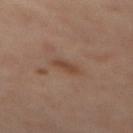| field | value |
|---|---|
| biopsy status | catalogued during a skin exam; not biopsied |
| image source | 15 mm crop, total-body photography |
| subject | male, approximately 55 years of age |
| TBP lesion metrics | an automated nevus-likeness rating near 0 out of 100 |
| illumination | cross-polarized |
| body site | the mid back |